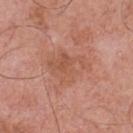A close-up tile cropped from a whole-body skin photograph, about 15 mm across. A male patient, aged approximately 70. From the chest.